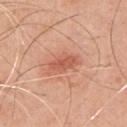Assessment:
Part of a total-body skin-imaging series; this lesion was reviewed on a skin check and was not flagged for biopsy.
Background:
Cropped from a whole-body photographic skin survey; the tile spans about 15 mm. The lesion is on the chest. This is a white-light tile. The patient is a male aged 48–52.